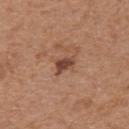The lesion was tiled from a total-body skin photograph and was not biopsied.